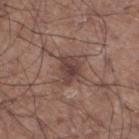Assessment:
Recorded during total-body skin imaging; not selected for excision or biopsy.
Context:
Automated image analysis of the tile measured a lesion color around L≈41 a*≈18 b*≈21 in CIELAB, a lesion–skin lightness drop of about 9, and a normalized lesion–skin contrast near 8. It also reported a classifier nevus-likeness of about 50/100. The lesion is on the left lower leg. A 15 mm close-up extracted from a 3D total-body photography capture. A male subject, about 60 years old.